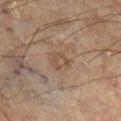The lesion was photographed on a routine skin check and not biopsied; there is no pathology result. Imaged with cross-polarized lighting. An algorithmic analysis of the crop reported a shape eccentricity near 0.4 and a symmetry-axis asymmetry near 0.3. The software also gave an average lesion color of about L≈36 a*≈12 b*≈22 (CIELAB) and roughly 5 lightness units darker than nearby skin. The analysis additionally found border irregularity of about 3 on a 0–10 scale, a color-variation rating of about 2/10, and peripheral color asymmetry of about 0.5. Measured at roughly 2.5 mm in maximum diameter. A 15 mm close-up extracted from a 3D total-body photography capture. Located on the left leg. A male patient aged approximately 60.